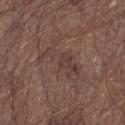Q: Was a biopsy performed?
A: imaged on a skin check; not biopsied
Q: What is the lesion's diameter?
A: ≈6 mm
Q: Who is the patient?
A: male, aged approximately 65
Q: Automated lesion metrics?
A: a lesion area of about 12 mm² and an outline eccentricity of about 0.9 (0 = round, 1 = elongated); a border-irregularity index near 6/10, a color-variation rating of about 4.5/10, and radial color variation of about 1.5; a classifier nevus-likeness of about 0/100 and lesion-presence confidence of about 90/100
Q: What is the anatomic site?
A: the left upper arm
Q: How was this image acquired?
A: ~15 mm tile from a whole-body skin photo